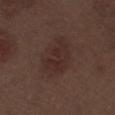This lesion was catalogued during total-body skin photography and was not selected for biopsy.
About 6 mm across.
Imaged with white-light lighting.
The lesion is on the right thigh.
The lesion-visualizer software estimated a lesion area of about 17 mm², an outline eccentricity of about 0.75 (0 = round, 1 = elongated), and a shape-asymmetry score of about 0.2 (0 = symmetric).
A 15 mm crop from a total-body photograph taken for skin-cancer surveillance.
The patient is a male about 70 years old.